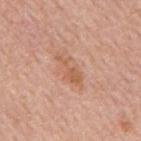Q: Was a biopsy performed?
A: total-body-photography surveillance lesion; no biopsy
Q: Lesion size?
A: ~4 mm (longest diameter)
Q: How was this image acquired?
A: ~15 mm tile from a whole-body skin photo
Q: Automated lesion metrics?
A: an area of roughly 4.5 mm², an outline eccentricity of about 0.9 (0 = round, 1 = elongated), and a shape-asymmetry score of about 0.55 (0 = symmetric); border irregularity of about 7.5 on a 0–10 scale, a within-lesion color-variation index near 1.5/10, and radial color variation of about 0.5
Q: Where on the body is the lesion?
A: the mid back
Q: Who is the patient?
A: male, about 55 years old
Q: Illumination type?
A: white-light illumination